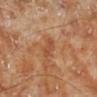image source: ~15 mm tile from a whole-body skin photo | patient: male, aged 68 to 72 | body site: the leg | lesion diameter: ≈3 mm | illumination: cross-polarized.